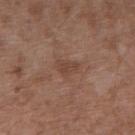| feature | finding |
|---|---|
| biopsy status | no biopsy performed (imaged during a skin exam) |
| automated lesion analysis | an area of roughly 3.5 mm², a shape eccentricity near 0.8, and a symmetry-axis asymmetry near 0.35 |
| subject | male, aged 48 to 52 |
| size | ≈2.5 mm |
| site | the right upper arm |
| acquisition | ~15 mm tile from a whole-body skin photo |
| lighting | white-light |